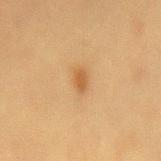Captured under cross-polarized illumination. Automated tile analysis of the lesion measured a lesion area of about 3 mm², an eccentricity of roughly 0.8, and two-axis asymmetry of about 0.2. And it measured an average lesion color of about L≈47 a*≈17 b*≈36 (CIELAB), a lesion–skin lightness drop of about 8, and a normalized lesion–skin contrast near 6.5. And it measured a border-irregularity rating of about 1.5/10, a color-variation rating of about 1/10, and a peripheral color-asymmetry measure near 0.5. A female subject aged around 55. On the lower back. Approximately 2.5 mm at its widest. A roughly 15 mm field-of-view crop from a total-body skin photograph.